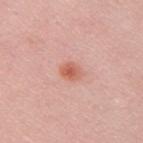Part of a total-body skin-imaging series; this lesion was reviewed on a skin check and was not flagged for biopsy.
The lesion's longest dimension is about 2.5 mm.
Imaged with white-light lighting.
The total-body-photography lesion software estimated a border-irregularity index near 2/10, a within-lesion color-variation index near 5/10, and a peripheral color-asymmetry measure near 1.5. The analysis additionally found a nevus-likeness score of about 75/100 and a lesion-detection confidence of about 100/100.
The patient is a male aged approximately 30.
This image is a 15 mm lesion crop taken from a total-body photograph.
Located on the arm.